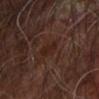<record>
  <site>arm</site>
  <patient>
    <sex>male</sex>
    <age_approx>65</age_approx>
  </patient>
  <image>
    <source>total-body photography crop</source>
    <field_of_view_mm>15</field_of_view_mm>
  </image>
</record>